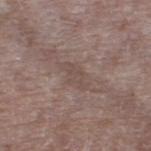  biopsy_status: not biopsied; imaged during a skin examination
  lighting: white-light
  patient:
    sex: male
    age_approx: 70
  site: left thigh
  image:
    source: total-body photography crop
    field_of_view_mm: 15
  lesion_size:
    long_diameter_mm_approx: 3.5